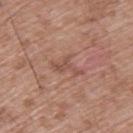Part of a total-body skin-imaging series; this lesion was reviewed on a skin check and was not flagged for biopsy. A male patient, roughly 50 years of age. Located on the upper back. A 15 mm crop from a total-body photograph taken for skin-cancer surveillance.